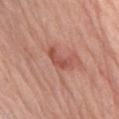The lesion was tiled from a total-body skin photograph and was not biopsied.
An algorithmic analysis of the crop reported an area of roughly 4.5 mm², an eccentricity of roughly 0.9, and two-axis asymmetry of about 0.4. And it measured an automated nevus-likeness rating near 65 out of 100 and a lesion-detection confidence of about 100/100.
Imaged with white-light lighting.
The lesion's longest dimension is about 3.5 mm.
The lesion is located on the right upper arm.
A 15 mm crop from a total-body photograph taken for skin-cancer surveillance.
A male patient in their mid-70s.